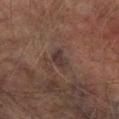- notes · total-body-photography surveillance lesion; no biopsy
- image · ~15 mm tile from a whole-body skin photo
- body site · the right lower leg
- lighting · cross-polarized
- patient · male, aged 73 to 77
- image-analysis metrics · an average lesion color of about L≈27 a*≈12 b*≈15 (CIELAB) and a normalized lesion–skin contrast near 8.5; a color-variation rating of about 2/10 and radial color variation of about 0.5